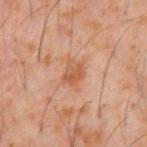Impression: This lesion was catalogued during total-body skin photography and was not selected for biopsy. Image and clinical context: Cropped from a whole-body photographic skin survey; the tile spans about 15 mm. A male patient roughly 60 years of age. The recorded lesion diameter is about 3 mm. The lesion is on the abdomen.